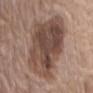biopsy status: catalogued during a skin exam; not biopsied | acquisition: ~15 mm crop, total-body skin-cancer survey | patient: female, in their mid-70s | lighting: white-light illumination | body site: the abdomen.